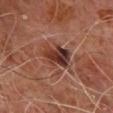Imaged during a routine full-body skin examination; the lesion was not biopsied and no histopathology is available.
A 15 mm crop from a total-body photograph taken for skin-cancer surveillance.
On the chest.
The lesion's longest dimension is about 4.5 mm.
The tile uses cross-polarized illumination.
A male subject aged 63–67.
An algorithmic analysis of the crop reported a mean CIELAB color near L≈35 a*≈23 b*≈24, a lesion–skin lightness drop of about 12, and a lesion-to-skin contrast of about 10 (normalized; higher = more distinct). The software also gave a within-lesion color-variation index near 9/10 and peripheral color asymmetry of about 3. It also reported a nevus-likeness score of about 0/100 and a lesion-detection confidence of about 100/100.